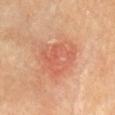Imaged during a routine full-body skin examination; the lesion was not biopsied and no histopathology is available.
A close-up tile cropped from a whole-body skin photograph, about 15 mm across.
The lesion is on the head or neck.
The tile uses cross-polarized illumination.
A female subject in their mid- to late 70s.
The lesion-visualizer software estimated a mean CIELAB color near L≈61 a*≈29 b*≈35, about 8 CIELAB-L* units darker than the surrounding skin, and a normalized border contrast of about 5. And it measured a classifier nevus-likeness of about 5/100 and a detector confidence of about 100 out of 100 that the crop contains a lesion.
Approximately 5 mm at its widest.